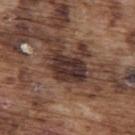{
  "biopsy_status": "not biopsied; imaged during a skin examination",
  "image": {
    "source": "total-body photography crop",
    "field_of_view_mm": 15
  },
  "lighting": "white-light",
  "lesion_size": {
    "long_diameter_mm_approx": 5.0
  },
  "patient": {
    "sex": "male",
    "age_approx": 75
  },
  "site": "upper back"
}